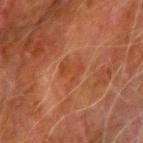notes=no biopsy performed (imaged during a skin exam)
subject=male, roughly 80 years of age
anatomic site=the left upper arm
lighting=cross-polarized
image=~15 mm crop, total-body skin-cancer survey
automated lesion analysis=an eccentricity of roughly 0.45